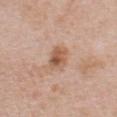site: chest
lesion_size:
  long_diameter_mm_approx: 3.0
lighting: white-light
image:
  source: total-body photography crop
  field_of_view_mm: 15
automated_metrics:
  nevus_likeness_0_100: 30
  lesion_detection_confidence_0_100: 100
patient:
  sex: female
  age_approx: 50